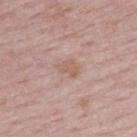A roughly 15 mm field-of-view crop from a total-body skin photograph. A female subject, approximately 50 years of age. Automated image analysis of the tile measured an area of roughly 3.5 mm² and a shape-asymmetry score of about 0.4 (0 = symmetric). The software also gave a lesion color around L≈59 a*≈19 b*≈26 in CIELAB, about 7 CIELAB-L* units darker than the surrounding skin, and a normalized lesion–skin contrast near 5.5. This is a white-light tile. Measured at roughly 2.5 mm in maximum diameter. The lesion is located on the upper back.